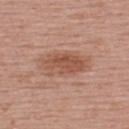workup: catalogued during a skin exam; not biopsied | image source: total-body-photography crop, ~15 mm field of view | site: the upper back | lighting: white-light | automated lesion analysis: a mean CIELAB color near L≈53 a*≈22 b*≈30, about 9 CIELAB-L* units darker than the surrounding skin, and a lesion-to-skin contrast of about 7 (normalized; higher = more distinct); a border-irregularity index near 3/10, a within-lesion color-variation index near 4/10, and peripheral color asymmetry of about 1.5; an automated nevus-likeness rating near 60 out of 100 | subject: female, roughly 55 years of age.